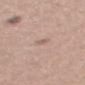biopsy status: no biopsy performed (imaged during a skin exam)
lesion diameter: about 1 mm
site: the right thigh
patient: female, approximately 30 years of age
image source: ~15 mm tile from a whole-body skin photo